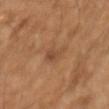Impression:
The lesion was tiled from a total-body skin photograph and was not biopsied.
Image and clinical context:
A male patient roughly 65 years of age. A 15 mm crop from a total-body photograph taken for skin-cancer surveillance.